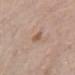Impression: The lesion was tiled from a total-body skin photograph and was not biopsied. Image and clinical context: The lesion's longest dimension is about 2.5 mm. Automated image analysis of the tile measured an average lesion color of about L≈56 a*≈19 b*≈28 (CIELAB), about 9 CIELAB-L* units darker than the surrounding skin, and a normalized border contrast of about 6.5. A female patient approximately 70 years of age. Imaged with white-light lighting. Cropped from a total-body skin-imaging series; the visible field is about 15 mm. From the right forearm.